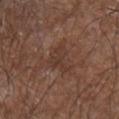notes=imaged on a skin check; not biopsied | image=total-body-photography crop, ~15 mm field of view | patient=male, aged approximately 85 or older | site=the left forearm.